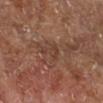This lesion was catalogued during total-body skin photography and was not selected for biopsy. The lesion is on the right lower leg. The subject is aged around 65. The total-body-photography lesion software estimated a lesion area of about 3.5 mm² and an eccentricity of roughly 0.85. And it measured roughly 6 lightness units darker than nearby skin. Captured under cross-polarized illumination. A lesion tile, about 15 mm wide, cut from a 3D total-body photograph.